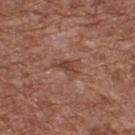{"biopsy_status": "not biopsied; imaged during a skin examination", "patient": {"sex": "male", "age_approx": 75}, "image": {"source": "total-body photography crop", "field_of_view_mm": 15}, "lesion_size": {"long_diameter_mm_approx": 3.0}, "site": "back", "lighting": "white-light", "automated_metrics": {"area_mm2_approx": 4.0, "shape_asymmetry": 0.4, "border_irregularity_0_10": 5.0, "color_variation_0_10": 1.0, "peripheral_color_asymmetry": 0.5, "lesion_detection_confidence_0_100": 100}}